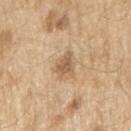Notes:
– biopsy status — no biopsy performed (imaged during a skin exam)